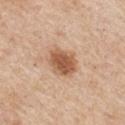Part of a total-body skin-imaging series; this lesion was reviewed on a skin check and was not flagged for biopsy.